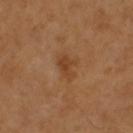biopsy status=no biopsy performed (imaged during a skin exam) | lighting=cross-polarized | image=15 mm crop, total-body photography | location=the head or neck | subject=male, aged around 50 | size=about 3.5 mm.